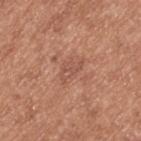No biopsy was performed on this lesion — it was imaged during a full skin examination and was not determined to be concerning. The total-body-photography lesion software estimated a lesion area of about 4 mm² and a symmetry-axis asymmetry near 0.45. It also reported a classifier nevus-likeness of about 0/100 and a lesion-detection confidence of about 100/100. The lesion is on the back. Captured under white-light illumination. A 15 mm close-up tile from a total-body photography series done for melanoma screening. The subject is a male aged 53 to 57.